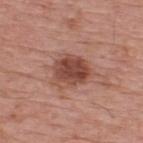follow-up=catalogued during a skin exam; not biopsied
patient=male, roughly 75 years of age
body site=the upper back
acquisition=~15 mm crop, total-body skin-cancer survey